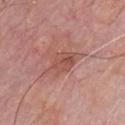Located on the chest.
A male subject in their 60s.
This is a white-light tile.
A 15 mm close-up extracted from a 3D total-body photography capture.
About 4 mm across.